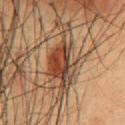Case summary:
– notes — no biopsy performed (imaged during a skin exam)
– anatomic site — the chest
– patient — male, in their 50s
– image source — ~15 mm tile from a whole-body skin photo